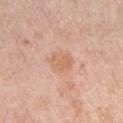The lesion was tiled from a total-body skin photograph and was not biopsied.
Imaged with white-light lighting.
The lesion is on the left upper arm.
A 15 mm close-up tile from a total-body photography series done for melanoma screening.
About 3.5 mm across.
A female patient roughly 65 years of age.